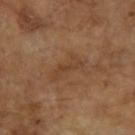Q: Was a biopsy performed?
A: catalogued during a skin exam; not biopsied
Q: How was the tile lit?
A: cross-polarized illumination
Q: What is the imaging modality?
A: ~15 mm tile from a whole-body skin photo
Q: Lesion location?
A: the right forearm
Q: How large is the lesion?
A: ~4 mm (longest diameter)
Q: Patient demographics?
A: female, in their 70s